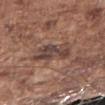Background:
Measured at roughly 4.5 mm in maximum diameter. From the right upper arm. An algorithmic analysis of the crop reported a lesion area of about 12 mm², a shape eccentricity near 0.75, and a shape-asymmetry score of about 0.25 (0 = symmetric). The analysis additionally found a lesion-detection confidence of about 70/100. Captured under white-light illumination. The subject is a male approximately 75 years of age. A lesion tile, about 15 mm wide, cut from a 3D total-body photograph.